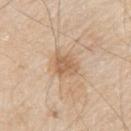Findings:
• workup — imaged on a skin check; not biopsied
• automated metrics — a normalized lesion–skin contrast near 6.5
• image — ~15 mm tile from a whole-body skin photo
• site — the right upper arm
• subject — male, approximately 80 years of age
• illumination — white-light illumination